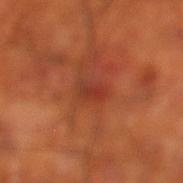Q: Was a biopsy performed?
A: no biopsy performed (imaged during a skin exam)
Q: Where on the body is the lesion?
A: the left lower leg
Q: Patient demographics?
A: male, aged approximately 70
Q: How was this image acquired?
A: ~15 mm tile from a whole-body skin photo